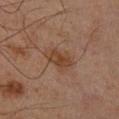workup: total-body-photography surveillance lesion; no biopsy | subject: male, approximately 65 years of age | location: the leg | acquisition: ~15 mm crop, total-body skin-cancer survey.